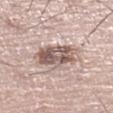<lesion>
<biopsy_status>not biopsied; imaged during a skin examination</biopsy_status>
<site>leg</site>
<patient>
  <sex>male</sex>
  <age_approx>70</age_approx>
</patient>
<lesion_size>
  <long_diameter_mm_approx>5.5</long_diameter_mm_approx>
</lesion_size>
<automated_metrics>
  <area_mm2_approx>16.0</area_mm2_approx>
  <eccentricity>0.7</eccentricity>
  <shape_asymmetry>0.2</shape_asymmetry>
  <border_irregularity_0_10>3.0</border_irregularity_0_10>
  <color_variation_0_10>8.5</color_variation_0_10>
  <peripheral_color_asymmetry>3.5</peripheral_color_asymmetry>
</automated_metrics>
<lighting>white-light</lighting>
<image>
  <source>total-body photography crop</source>
  <field_of_view_mm>15</field_of_view_mm>
</image>
</lesion>